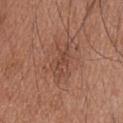{"biopsy_status": "not biopsied; imaged during a skin examination", "site": "upper back", "lighting": "white-light", "lesion_size": {"long_diameter_mm_approx": 5.0}, "patient": {"sex": "male", "age_approx": 45}, "image": {"source": "total-body photography crop", "field_of_view_mm": 15}}